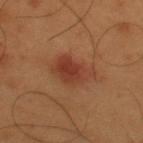notes = no biopsy performed (imaged during a skin exam)
imaging modality = total-body-photography crop, ~15 mm field of view
body site = the upper back
patient = male, aged 53–57